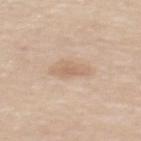Case summary:
* biopsy status: imaged on a skin check; not biopsied
* lighting: white-light
* image source: 15 mm crop, total-body photography
* location: the upper back
* subject: female, aged 63 to 67